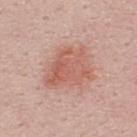Assessment: Imaged during a routine full-body skin examination; the lesion was not biopsied and no histopathology is available. Acquisition and patient details: Captured under white-light illumination. A male patient roughly 40 years of age. The lesion is located on the upper back. This image is a 15 mm lesion crop taken from a total-body photograph. The lesion-visualizer software estimated a lesion area of about 17 mm², an eccentricity of roughly 0.6, and a symmetry-axis asymmetry near 0.3. And it measured an average lesion color of about L≈59 a*≈24 b*≈28 (CIELAB), a lesion–skin lightness drop of about 9, and a normalized lesion–skin contrast near 6.5.